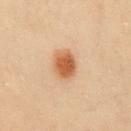image source: 15 mm crop, total-body photography
site: the front of the torso
lesion size: about 3.5 mm
patient: female, aged around 40